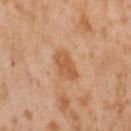Clinical impression:
This lesion was catalogued during total-body skin photography and was not selected for biopsy.
Background:
The lesion is located on the left thigh. The recorded lesion diameter is about 3.5 mm. Captured under cross-polarized illumination. A female patient, approximately 55 years of age. A 15 mm close-up extracted from a 3D total-body photography capture.